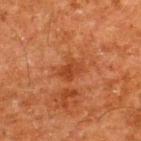Imaged during a routine full-body skin examination; the lesion was not biopsied and no histopathology is available. A male patient, aged 58 to 62. From the upper back. A 15 mm crop from a total-body photograph taken for skin-cancer surveillance. The lesion-visualizer software estimated a footprint of about 4.5 mm², an eccentricity of roughly 0.7, and a symmetry-axis asymmetry near 0.35. The software also gave a lesion color around L≈34 a*≈25 b*≈33 in CIELAB, roughly 7 lightness units darker than nearby skin, and a normalized border contrast of about 6.5. The analysis additionally found a border-irregularity rating of about 3/10, internal color variation of about 2.5 on a 0–10 scale, and a peripheral color-asymmetry measure near 1. The tile uses cross-polarized illumination.